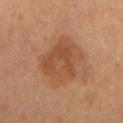patient: male, in their mid- to late 50s | tile lighting: cross-polarized illumination | site: the chest | diameter: ~6 mm (longest diameter) | imaging modality: ~15 mm crop, total-body skin-cancer survey | automated lesion analysis: a footprint of about 21 mm², an outline eccentricity of about 0.7 (0 = round, 1 = elongated), and a shape-asymmetry score of about 0.2 (0 = symmetric); a mean CIELAB color near L≈45 a*≈21 b*≈31, a lesion–skin lightness drop of about 8, and a normalized lesion–skin contrast near 6.5.